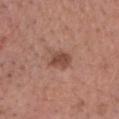Impression:
The lesion was tiled from a total-body skin photograph and was not biopsied.
Acquisition and patient details:
This image is a 15 mm lesion crop taken from a total-body photograph. A male patient aged 53–57.